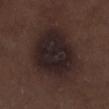The lesion was tiled from a total-body skin photograph and was not biopsied.
Longest diameter approximately 8 mm.
A male patient, aged approximately 70.
A roughly 15 mm field-of-view crop from a total-body skin photograph.
The tile uses white-light illumination.
The lesion is on the left lower leg.